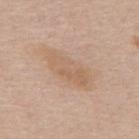<record>
  <biopsy_status>not biopsied; imaged during a skin examination</biopsy_status>
  <site>upper back</site>
  <patient>
    <sex>male</sex>
    <age_approx>60</age_approx>
  </patient>
  <image>
    <source>total-body photography crop</source>
    <field_of_view_mm>15</field_of_view_mm>
  </image>
  <lighting>white-light</lighting>
  <lesion_size>
    <long_diameter_mm_approx>7.0</long_diameter_mm_approx>
  </lesion_size>
</record>